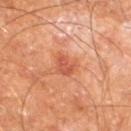{"biopsy_status": "not biopsied; imaged during a skin examination", "patient": {"sex": "male", "age_approx": 65}, "automated_metrics": {"border_irregularity_0_10": 3.0, "color_variation_0_10": 2.5, "peripheral_color_asymmetry": 1.0}, "lesion_size": {"long_diameter_mm_approx": 3.0}, "site": "right thigh", "lighting": "cross-polarized", "image": {"source": "total-body photography crop", "field_of_view_mm": 15}}